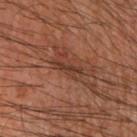Captured during whole-body skin photography for melanoma surveillance; the lesion was not biopsied. The patient is a male about 60 years old. The lesion's longest dimension is about 3.5 mm. On the right forearm. Automated image analysis of the tile measured a footprint of about 3.5 mm² and an outline eccentricity of about 0.95 (0 = round, 1 = elongated). The software also gave an automated nevus-likeness rating near 0 out of 100 and a lesion-detection confidence of about 50/100. A roughly 15 mm field-of-view crop from a total-body skin photograph. Captured under cross-polarized illumination.